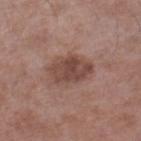Automated image analysis of the tile measured an average lesion color of about L≈45 a*≈20 b*≈24 (CIELAB), about 10 CIELAB-L* units darker than the surrounding skin, and a normalized lesion–skin contrast near 8. The software also gave a nevus-likeness score of about 45/100 and a lesion-detection confidence of about 100/100.
A 15 mm close-up tile from a total-body photography series done for melanoma screening.
The recorded lesion diameter is about 4.5 mm.
The lesion is located on the leg.
This is a white-light tile.
A male subject, aged around 55.